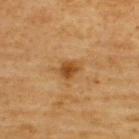Captured during whole-body skin photography for melanoma surveillance; the lesion was not biopsied.